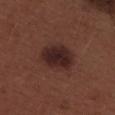Captured under white-light illumination. Located on the upper back. This image is a 15 mm lesion crop taken from a total-body photograph. The patient is a male aged 28–32. Longest diameter approximately 5 mm.